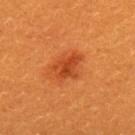follow-up: total-body-photography surveillance lesion; no biopsy | subject: female, aged approximately 30 | body site: the upper back | lighting: cross-polarized illumination | image: ~15 mm tile from a whole-body skin photo | lesion size: ≈3.5 mm.